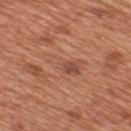Impression: Part of a total-body skin-imaging series; this lesion was reviewed on a skin check and was not flagged for biopsy. Context: The lesion is on the upper back. A lesion tile, about 15 mm wide, cut from a 3D total-body photograph. The tile uses white-light illumination. The subject is a male in their mid- to late 60s.